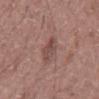Case summary:
* biopsy status: no biopsy performed (imaged during a skin exam)
* illumination: white-light
* site: the chest
* subject: male, roughly 60 years of age
* acquisition: 15 mm crop, total-body photography